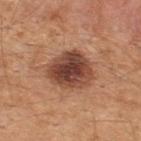{
  "biopsy_status": "not biopsied; imaged during a skin examination",
  "lighting": "white-light",
  "patient": {
    "sex": "male",
    "age_approx": 45
  },
  "site": "back",
  "image": {
    "source": "total-body photography crop",
    "field_of_view_mm": 15
  },
  "lesion_size": {
    "long_diameter_mm_approx": 5.5
  }
}